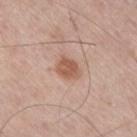Imaged during a routine full-body skin examination; the lesion was not biopsied and no histopathology is available. A male subject roughly 75 years of age. Longest diameter approximately 3 mm. On the right thigh. This is a white-light tile. This image is a 15 mm lesion crop taken from a total-body photograph. Automated image analysis of the tile measured a lesion area of about 6.5 mm², an outline eccentricity of about 0.6 (0 = round, 1 = elongated), and a symmetry-axis asymmetry near 0.2. It also reported a mean CIELAB color near L≈57 a*≈21 b*≈29, about 10 CIELAB-L* units darker than the surrounding skin, and a normalized lesion–skin contrast near 7.5. The software also gave border irregularity of about 1.5 on a 0–10 scale, a color-variation rating of about 2.5/10, and radial color variation of about 1.